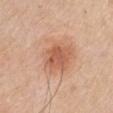Imaged during a routine full-body skin examination; the lesion was not biopsied and no histopathology is available.
This is a white-light tile.
A male patient approximately 65 years of age.
The recorded lesion diameter is about 6.5 mm.
This image is a 15 mm lesion crop taken from a total-body photograph.
The lesion-visualizer software estimated a lesion area of about 32 mm² and an eccentricity of roughly 0.35. The software also gave a border-irregularity rating of about 2/10, a within-lesion color-variation index near 6/10, and a peripheral color-asymmetry measure near 1.5. It also reported a nevus-likeness score of about 80/100 and a lesion-detection confidence of about 100/100.
From the right upper arm.